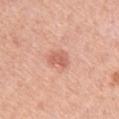No biopsy was performed on this lesion — it was imaged during a full skin examination and was not determined to be concerning.